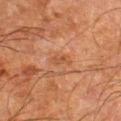<lesion>
  <biopsy_status>not biopsied; imaged during a skin examination</biopsy_status>
</lesion>